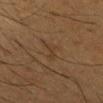Case summary:
• lighting: cross-polarized
• site: the head or neck
• lesion size: ≈3 mm
• patient: male, aged approximately 40
• image source: total-body-photography crop, ~15 mm field of view